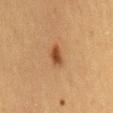Approximately 3 mm at its widest.
Imaged with cross-polarized lighting.
A female subject about 40 years old.
A lesion tile, about 15 mm wide, cut from a 3D total-body photograph.
Located on the back.